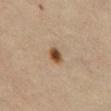Q: Was this lesion biopsied?
A: catalogued during a skin exam; not biopsied
Q: What did automated image analysis measure?
A: a footprint of about 3.5 mm² and a shape eccentricity near 0.7; a mean CIELAB color near L≈46 a*≈19 b*≈33, about 15 CIELAB-L* units darker than the surrounding skin, and a lesion-to-skin contrast of about 11.5 (normalized; higher = more distinct); a within-lesion color-variation index near 4/10 and peripheral color asymmetry of about 1; an automated nevus-likeness rating near 100 out of 100 and a lesion-detection confidence of about 100/100
Q: What lighting was used for the tile?
A: cross-polarized
Q: Where on the body is the lesion?
A: the abdomen
Q: What are the patient's age and sex?
A: male, aged 63 to 67
Q: How large is the lesion?
A: about 2.5 mm
Q: What is the imaging modality?
A: ~15 mm crop, total-body skin-cancer survey